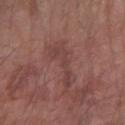workup: catalogued during a skin exam; not biopsied | acquisition: ~15 mm tile from a whole-body skin photo | subject: male, about 80 years old | anatomic site: the arm | lesion diameter: about 5 mm | TBP lesion metrics: border irregularity of about 7.5 on a 0–10 scale and a peripheral color-asymmetry measure near 0.5; a nevus-likeness score of about 0/100 | illumination: white-light.